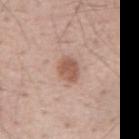The lesion is on the mid back. Cropped from a whole-body photographic skin survey; the tile spans about 15 mm. Captured under white-light illumination. The patient is a male approximately 55 years of age.